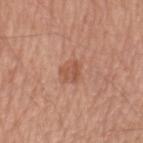Notes:
• follow-up — catalogued during a skin exam; not biopsied
• image — ~15 mm crop, total-body skin-cancer survey
• anatomic site — the left upper arm
• subject — male, aged approximately 70